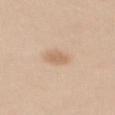biopsy status = total-body-photography surveillance lesion; no biopsy | tile lighting = white-light illumination | lesion diameter = ≈3 mm | image = total-body-photography crop, ~15 mm field of view | image-analysis metrics = border irregularity of about 2.5 on a 0–10 scale, internal color variation of about 1 on a 0–10 scale, and peripheral color asymmetry of about 0.5 | anatomic site = the mid back | subject = female, aged 38–42.